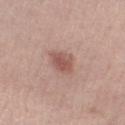This is a white-light tile.
A 15 mm close-up extracted from a 3D total-body photography capture.
The subject is a female roughly 20 years of age.
The lesion's longest dimension is about 2.5 mm.
Automated image analysis of the tile measured roughly 10 lightness units darker than nearby skin and a normalized lesion–skin contrast near 7.
Located on the left lower leg.